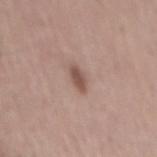Captured during whole-body skin photography for melanoma surveillance; the lesion was not biopsied.
A male patient, aged 53 to 57.
Captured under white-light illumination.
This image is a 15 mm lesion crop taken from a total-body photograph.
Longest diameter approximately 3 mm.